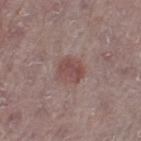Captured during whole-body skin photography for melanoma surveillance; the lesion was not biopsied.
Located on the left thigh.
Cropped from a whole-body photographic skin survey; the tile spans about 15 mm.
The subject is a male about 75 years old.